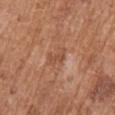Findings:
• biopsy status: catalogued during a skin exam; not biopsied
• image source: 15 mm crop, total-body photography
• image-analysis metrics: an area of roughly 4 mm² and a symmetry-axis asymmetry near 0.4; an average lesion color of about L≈51 a*≈24 b*≈33 (CIELAB) and roughly 7 lightness units darker than nearby skin; an automated nevus-likeness rating near 0 out of 100 and lesion-presence confidence of about 100/100
• subject: female, in their mid- to late 70s
• anatomic site: the arm
• size: about 3.5 mm
• lighting: white-light illumination